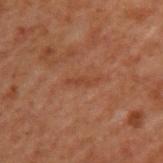workup = total-body-photography surveillance lesion; no biopsy | image source = ~15 mm crop, total-body skin-cancer survey | location = the upper back | subject = male, aged around 70 | tile lighting = cross-polarized illumination.